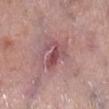Clinical impression:
This lesion was catalogued during total-body skin photography and was not selected for biopsy.